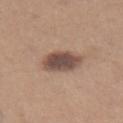Findings:
- follow-up — imaged on a skin check; not biopsied
- body site — the left thigh
- subject — female, about 40 years old
- lighting — white-light
- acquisition — ~15 mm crop, total-body skin-cancer survey
- image-analysis metrics — an area of roughly 10 mm², an outline eccentricity of about 0.65 (0 = round, 1 = elongated), and a shape-asymmetry score of about 0.2 (0 = symmetric); an average lesion color of about L≈48 a*≈17 b*≈23 (CIELAB), roughly 14 lightness units darker than nearby skin, and a normalized lesion–skin contrast near 10.5; a within-lesion color-variation index near 4/10 and radial color variation of about 1